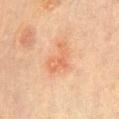  biopsy_status: not biopsied; imaged during a skin examination
  automated_metrics:
    area_mm2_approx: 7.0
    eccentricity: 0.8
    cielab_L: 57
    cielab_a: 24
    cielab_b: 33
    vs_skin_darker_L: 7.0
    vs_skin_contrast_norm: 5.5
    border_irregularity_0_10: 6.0
    color_variation_0_10: 3.5
    peripheral_color_asymmetry: 1.5
  lighting: cross-polarized
  image:
    source: total-body photography crop
    field_of_view_mm: 15
  lesion_size:
    long_diameter_mm_approx: 4.0
  patient:
    sex: female
    age_approx: 65
  site: abdomen